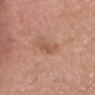{"biopsy_status": "not biopsied; imaged during a skin examination", "patient": {"sex": "male", "age_approx": 65}, "lighting": "white-light", "image": {"source": "total-body photography crop", "field_of_view_mm": 15}, "site": "head or neck", "lesion_size": {"long_diameter_mm_approx": 2.5}}